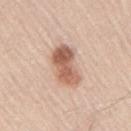biopsy status — total-body-photography surveillance lesion; no biopsy
tile lighting — white-light illumination
image — 15 mm crop, total-body photography
subject — male, in their mid- to late 40s
image-analysis metrics — a lesion color around L≈60 a*≈21 b*≈30 in CIELAB, about 14 CIELAB-L* units darker than the surrounding skin, and a normalized border contrast of about 9; a border-irregularity rating of about 3.5/10, a color-variation rating of about 5.5/10, and radial color variation of about 2
site — the right upper arm
size — ~5.5 mm (longest diameter)